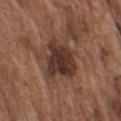Recorded during total-body skin imaging; not selected for excision or biopsy.
A roughly 15 mm field-of-view crop from a total-body skin photograph.
From the left upper arm.
A male patient aged around 75.